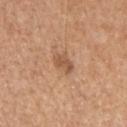On the head or neck. This is a white-light tile. Cropped from a whole-body photographic skin survey; the tile spans about 15 mm. A male subject approximately 60 years of age.